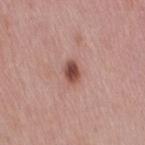Recorded during total-body skin imaging; not selected for excision or biopsy. Captured under white-light illumination. The subject is a female approximately 40 years of age. An algorithmic analysis of the crop reported a mean CIELAB color near L≈48 a*≈24 b*≈25 and a lesion-to-skin contrast of about 10.5 (normalized; higher = more distinct). The software also gave a border-irregularity index near 2/10 and a color-variation rating of about 3.5/10. The software also gave a classifier nevus-likeness of about 95/100 and lesion-presence confidence of about 100/100. Longest diameter approximately 2.5 mm. A close-up tile cropped from a whole-body skin photograph, about 15 mm across. On the right thigh.